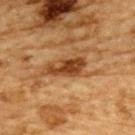Findings:
- notes: no biopsy performed (imaged during a skin exam)
- body site: the upper back
- automated metrics: a lesion area of about 7.5 mm² and an outline eccentricity of about 0.9 (0 = round, 1 = elongated)
- acquisition: ~15 mm crop, total-body skin-cancer survey
- subject: male, aged approximately 85 or older
- tile lighting: cross-polarized